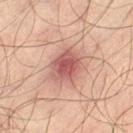A close-up tile cropped from a whole-body skin photograph, about 15 mm across. The patient is a male in their mid- to late 30s. Longest diameter approximately 4.5 mm. From the left thigh. This is a cross-polarized tile.